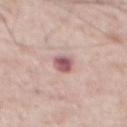{"biopsy_status": "not biopsied; imaged during a skin examination", "site": "abdomen", "lighting": "white-light", "image": {"source": "total-body photography crop", "field_of_view_mm": 15}, "patient": {"sex": "male", "age_approx": 55}}